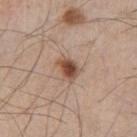follow-up — imaged on a skin check; not biopsied
subject — male, aged approximately 60
anatomic site — the left thigh
imaging modality — ~15 mm tile from a whole-body skin photo